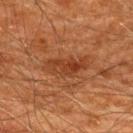Assessment:
Imaged during a routine full-body skin examination; the lesion was not biopsied and no histopathology is available.
Context:
A lesion tile, about 15 mm wide, cut from a 3D total-body photograph. About 5.5 mm across. The lesion is located on the upper back. A male patient, approximately 60 years of age. The lesion-visualizer software estimated a mean CIELAB color near L≈31 a*≈21 b*≈29, a lesion–skin lightness drop of about 7, and a normalized lesion–skin contrast near 6.5. The software also gave a within-lesion color-variation index near 4/10 and a peripheral color-asymmetry measure near 1.5. The software also gave a nevus-likeness score of about 15/100 and lesion-presence confidence of about 100/100.